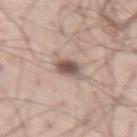Imaged during a routine full-body skin examination; the lesion was not biopsied and no histopathology is available.
Cropped from a total-body skin-imaging series; the visible field is about 15 mm.
The lesion is on the right thigh.
Captured under white-light illumination.
A male subject aged around 40.
Measured at roughly 3.5 mm in maximum diameter.